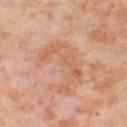Findings:
– biopsy status · catalogued during a skin exam; not biopsied
– image · ~15 mm tile from a whole-body skin photo
– location · the right thigh
– subject · female, aged approximately 55
– image-analysis metrics · a mean CIELAB color near L≈62 a*≈23 b*≈34, roughly 7 lightness units darker than nearby skin, and a normalized border contrast of about 5.5; a border-irregularity rating of about 10/10 and peripheral color asymmetry of about 1; a nevus-likeness score of about 0/100 and a detector confidence of about 100 out of 100 that the crop contains a lesion